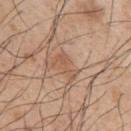Case summary:
- workup — no biopsy performed (imaged during a skin exam)
- imaging modality — total-body-photography crop, ~15 mm field of view
- anatomic site — the left upper arm
- tile lighting — white-light
- lesion size — about 3.5 mm
- patient — male, aged approximately 65